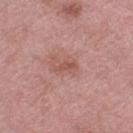Captured during whole-body skin photography for melanoma surveillance; the lesion was not biopsied. A female patient, aged around 70. Located on the left thigh. Longest diameter approximately 2.5 mm. This image is a 15 mm lesion crop taken from a total-body photograph. The total-body-photography lesion software estimated a footprint of about 2 mm², an outline eccentricity of about 0.9 (0 = round, 1 = elongated), and two-axis asymmetry of about 0.4. The software also gave border irregularity of about 4 on a 0–10 scale, a within-lesion color-variation index near 0/10, and peripheral color asymmetry of about 0. And it measured an automated nevus-likeness rating near 0 out of 100 and a lesion-detection confidence of about 100/100.